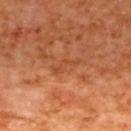Clinical impression: Captured during whole-body skin photography for melanoma surveillance; the lesion was not biopsied. Acquisition and patient details: Located on the upper back. This is a cross-polarized tile. Cropped from a whole-body photographic skin survey; the tile spans about 15 mm. A female patient, about 40 years old. The recorded lesion diameter is about 3.5 mm.